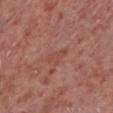{"biopsy_status": "not biopsied; imaged during a skin examination", "lesion_size": {"long_diameter_mm_approx": 3.0}, "patient": {"sex": "male", "age_approx": 55}, "image": {"source": "total-body photography crop", "field_of_view_mm": 15}, "site": "left lower leg"}